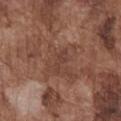Clinical impression: The lesion was photographed on a routine skin check and not biopsied; there is no pathology result. Context: A close-up tile cropped from a whole-body skin photograph, about 15 mm across. This is a white-light tile. On the chest. The patient is a male in their mid-70s. Automated tile analysis of the lesion measured a peripheral color-asymmetry measure near 0. And it measured a classifier nevus-likeness of about 0/100 and a detector confidence of about 95 out of 100 that the crop contains a lesion.